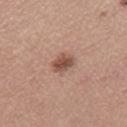workup: no biopsy performed (imaged during a skin exam) | anatomic site: the right thigh | lesion diameter: ~3 mm (longest diameter) | image: ~15 mm tile from a whole-body skin photo | image-analysis metrics: a border-irregularity index near 2/10 and a within-lesion color-variation index near 3.5/10; a nevus-likeness score of about 90/100 and a detector confidence of about 100 out of 100 that the crop contains a lesion | subject: female, aged approximately 45.